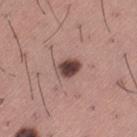workup: no biopsy performed (imaged during a skin exam) | anatomic site: the left thigh | subject: male, aged 43–47 | imaging modality: total-body-photography crop, ~15 mm field of view.